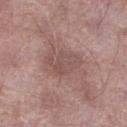This lesion was catalogued during total-body skin photography and was not selected for biopsy.
Measured at roughly 4.5 mm in maximum diameter.
From the leg.
The subject is a male aged 53–57.
Captured under white-light illumination.
Cropped from a whole-body photographic skin survey; the tile spans about 15 mm.
An algorithmic analysis of the crop reported a mean CIELAB color near L≈51 a*≈19 b*≈21, roughly 7 lightness units darker than nearby skin, and a normalized lesion–skin contrast near 5. The software also gave border irregularity of about 4 on a 0–10 scale and a within-lesion color-variation index near 2.5/10. The software also gave an automated nevus-likeness rating near 0 out of 100 and a lesion-detection confidence of about 100/100.